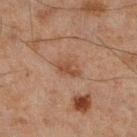Captured during whole-body skin photography for melanoma surveillance; the lesion was not biopsied. Located on the leg. A male subject, aged 43–47. Longest diameter approximately 3 mm. A close-up tile cropped from a whole-body skin photograph, about 15 mm across. The tile uses cross-polarized illumination. The total-body-photography lesion software estimated a lesion area of about 4.5 mm² and two-axis asymmetry of about 0.3. The analysis additionally found an automated nevus-likeness rating near 10 out of 100 and a lesion-detection confidence of about 100/100.